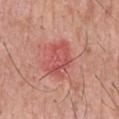The lesion is on the chest.
The subject is a male aged around 55.
A lesion tile, about 15 mm wide, cut from a 3D total-body photograph.